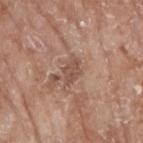Part of a total-body skin-imaging series; this lesion was reviewed on a skin check and was not flagged for biopsy. A female subject, about 75 years old. The lesion is on the right upper arm. A close-up tile cropped from a whole-body skin photograph, about 15 mm across.